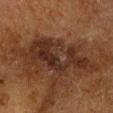biopsy status: catalogued during a skin exam; not biopsied | TBP lesion metrics: a mean CIELAB color near L≈24 a*≈16 b*≈22 and a normalized lesion–skin contrast near 9.5; border irregularity of about 7 on a 0–10 scale, a color-variation rating of about 5.5/10, and peripheral color asymmetry of about 2; an automated nevus-likeness rating near 15 out of 100 and a detector confidence of about 95 out of 100 that the crop contains a lesion | imaging modality: ~15 mm crop, total-body skin-cancer survey | lesion diameter: ≈8.5 mm | subject: male, aged around 60 | lighting: cross-polarized | site: the head or neck.